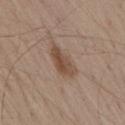Recorded during total-body skin imaging; not selected for excision or biopsy.
The total-body-photography lesion software estimated a lesion area of about 7.5 mm² and two-axis asymmetry of about 0.3. And it measured border irregularity of about 3.5 on a 0–10 scale, a color-variation rating of about 4/10, and a peripheral color-asymmetry measure near 1.5.
A male patient aged 53–57.
From the lower back.
Cropped from a whole-body photographic skin survey; the tile spans about 15 mm.
The recorded lesion diameter is about 4.5 mm.
The tile uses white-light illumination.